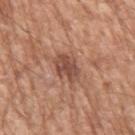Q: Is there a histopathology result?
A: total-body-photography surveillance lesion; no biopsy
Q: Patient demographics?
A: male, aged 63 to 67
Q: How was this image acquired?
A: ~15 mm tile from a whole-body skin photo
Q: What is the anatomic site?
A: the left upper arm